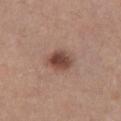{
  "biopsy_status": "not biopsied; imaged during a skin examination",
  "image": {
    "source": "total-body photography crop",
    "field_of_view_mm": 15
  },
  "site": "front of the torso",
  "automated_metrics": {
    "cielab_L": 45,
    "cielab_a": 20,
    "cielab_b": 25,
    "vs_skin_darker_L": 13.0,
    "vs_skin_contrast_norm": 9.5,
    "nevus_likeness_0_100": 95,
    "lesion_detection_confidence_0_100": 100
  },
  "lighting": "white-light",
  "patient": {
    "sex": "female",
    "age_approx": 55
  }
}